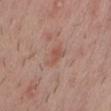notes: imaged on a skin check; not biopsied
automated lesion analysis: a footprint of about 4.5 mm² and a symmetry-axis asymmetry near 0.4; a border-irregularity rating of about 4/10 and a peripheral color-asymmetry measure near 1; a classifier nevus-likeness of about 0/100 and lesion-presence confidence of about 100/100
lighting: white-light illumination
image: 15 mm crop, total-body photography
site: the chest
patient: male, about 30 years old
size: about 3 mm